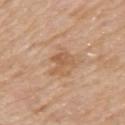This lesion was catalogued during total-body skin photography and was not selected for biopsy.
On the arm.
Longest diameter approximately 3.5 mm.
The tile uses white-light illumination.
The patient is a male about 75 years old.
A lesion tile, about 15 mm wide, cut from a 3D total-body photograph.